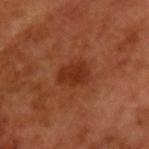  biopsy_status: not biopsied; imaged during a skin examination
  lesion_size:
    long_diameter_mm_approx: 3.0
  patient:
    sex: male
    age_approx: 65
  image:
    source: total-body photography crop
    field_of_view_mm: 15